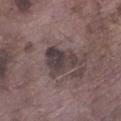{
  "biopsy_status": "not biopsied; imaged during a skin examination",
  "lighting": "white-light",
  "site": "left lower leg",
  "lesion_size": {
    "long_diameter_mm_approx": 4.5
  },
  "image": {
    "source": "total-body photography crop",
    "field_of_view_mm": 15
  },
  "patient": {
    "sex": "male",
    "age_approx": 75
  }
}